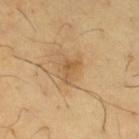Notes:
* location — the leg
* TBP lesion metrics — an average lesion color of about L≈57 a*≈18 b*≈39 (CIELAB) and a normalized border contrast of about 6; border irregularity of about 4.5 on a 0–10 scale and a color-variation rating of about 3/10; a detector confidence of about 100 out of 100 that the crop contains a lesion
* tile lighting — cross-polarized illumination
* image — ~15 mm tile from a whole-body skin photo
* subject — male, approximately 65 years of age